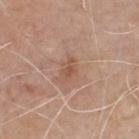Assessment:
Imaged during a routine full-body skin examination; the lesion was not biopsied and no histopathology is available.
Context:
Captured under white-light illumination. The lesion is located on the chest. A 15 mm close-up extracted from a 3D total-body photography capture. Longest diameter approximately 2.5 mm. A male patient aged around 75.